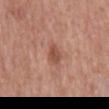The lesion was tiled from a total-body skin photograph and was not biopsied.
Approximately 2.5 mm at its widest.
Cropped from a total-body skin-imaging series; the visible field is about 15 mm.
From the mid back.
A male patient aged 63 to 67.
Automated image analysis of the tile measured border irregularity of about 2 on a 0–10 scale and a color-variation rating of about 2/10.
This is a white-light tile.